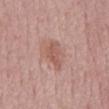Impression: No biopsy was performed on this lesion — it was imaged during a full skin examination and was not determined to be concerning. Clinical summary: Captured under white-light illumination. Cropped from a whole-body photographic skin survey; the tile spans about 15 mm. An algorithmic analysis of the crop reported a lesion color around L≈56 a*≈22 b*≈26 in CIELAB, about 8 CIELAB-L* units darker than the surrounding skin, and a normalized border contrast of about 6. The analysis additionally found a color-variation rating of about 2.5/10 and a peripheral color-asymmetry measure near 1. A male subject, about 60 years old. Located on the mid back. The lesion's longest dimension is about 4.5 mm.